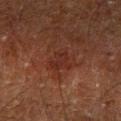Clinical impression: Captured during whole-body skin photography for melanoma surveillance; the lesion was not biopsied. Acquisition and patient details: Captured under cross-polarized illumination. The patient is a male aged around 60. Automated image analysis of the tile measured a lesion area of about 5.5 mm², a shape eccentricity near 0.6, and a shape-asymmetry score of about 0.4 (0 = symmetric). It also reported border irregularity of about 4.5 on a 0–10 scale, internal color variation of about 3 on a 0–10 scale, and radial color variation of about 1. The software also gave a classifier nevus-likeness of about 5/100 and a lesion-detection confidence of about 100/100. From the left forearm. The recorded lesion diameter is about 3 mm. A 15 mm close-up tile from a total-body photography series done for melanoma screening.